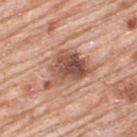biopsy status: imaged on a skin check; not biopsied | lighting: white-light illumination | location: the upper back | patient: male, aged around 80 | automated lesion analysis: a footprint of about 15 mm², a shape eccentricity near 0.7, and two-axis asymmetry of about 0.4; an average lesion color of about L≈53 a*≈22 b*≈28 (CIELAB), roughly 14 lightness units darker than nearby skin, and a lesion-to-skin contrast of about 9.5 (normalized; higher = more distinct); a border-irregularity index near 5.5/10, a within-lesion color-variation index near 8/10, and a peripheral color-asymmetry measure near 3 | image source: total-body-photography crop, ~15 mm field of view.